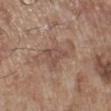lesion diameter = about 3 mm | patient = male, roughly 70 years of age | imaging modality = 15 mm crop, total-body photography | anatomic site = the left lower leg | illumination = white-light | image-analysis metrics = a border-irregularity index near 5/10, a within-lesion color-variation index near 3.5/10, and radial color variation of about 1.5.